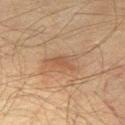The recorded lesion diameter is about 3.5 mm. Automated image analysis of the tile measured a lesion area of about 3.5 mm², a shape eccentricity near 0.9, and a symmetry-axis asymmetry near 0.4. It also reported about 6 CIELAB-L* units darker than the surrounding skin. A male patient aged 48 to 52. On the right thigh. A 15 mm close-up extracted from a 3D total-body photography capture. This is a cross-polarized tile.